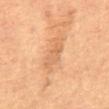Captured during whole-body skin photography for melanoma surveillance; the lesion was not biopsied. A female patient, roughly 70 years of age. Located on the abdomen. The lesion's longest dimension is about 4.5 mm. Cropped from a total-body skin-imaging series; the visible field is about 15 mm.